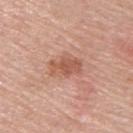biopsy status: total-body-photography surveillance lesion; no biopsy
location: the back
subject: male, in their 70s
diameter: ~4 mm (longest diameter)
lighting: white-light
image: ~15 mm tile from a whole-body skin photo